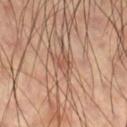biopsy_status: not biopsied; imaged during a skin examination
lighting: cross-polarized
site: right thigh
patient:
  sex: male
  age_approx: 60
image:
  source: total-body photography crop
  field_of_view_mm: 15